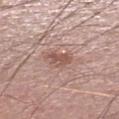| key | value |
|---|---|
| notes | total-body-photography surveillance lesion; no biopsy |
| automated metrics | a lesion-detection confidence of about 100/100 |
| image source | ~15 mm tile from a whole-body skin photo |
| illumination | white-light illumination |
| lesion size | about 3 mm |
| subject | male, roughly 55 years of age |
| anatomic site | the leg |